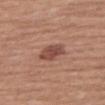Q: Is there a histopathology result?
A: total-body-photography surveillance lesion; no biopsy
Q: Patient demographics?
A: female, approximately 75 years of age
Q: Lesion location?
A: the right thigh
Q: What kind of image is this?
A: total-body-photography crop, ~15 mm field of view
Q: What lighting was used for the tile?
A: white-light